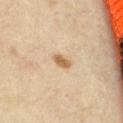tile lighting: cross-polarized illumination; anatomic site: the abdomen; lesion size: ≈2.5 mm; image: ~15 mm tile from a whole-body skin photo; subject: female, in their mid- to late 30s; automated lesion analysis: a lesion color around L≈60 a*≈16 b*≈37 in CIELAB, a lesion–skin lightness drop of about 12, and a normalized lesion–skin contrast near 9.